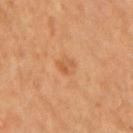image-analysis metrics=a border-irregularity rating of about 2.5/10, a color-variation rating of about 3/10, and radial color variation of about 1 | acquisition=~15 mm crop, total-body skin-cancer survey | diameter=about 2.5 mm | site=the arm | subject=male, in their mid-50s.